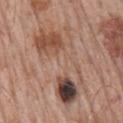biopsy_status: not biopsied; imaged during a skin examination
lesion_size:
  long_diameter_mm_approx: 10.5
site: mid back
image:
  source: total-body photography crop
  field_of_view_mm: 15
patient:
  sex: male
  age_approx: 75